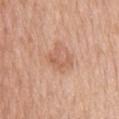Impression:
Imaged during a routine full-body skin examination; the lesion was not biopsied and no histopathology is available.